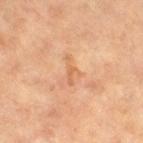This lesion was catalogued during total-body skin photography and was not selected for biopsy.